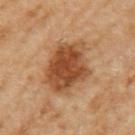Q: Was this lesion biopsied?
A: total-body-photography surveillance lesion; no biopsy
Q: Patient demographics?
A: female, approximately 60 years of age
Q: What is the anatomic site?
A: the left upper arm
Q: Lesion size?
A: about 6.5 mm
Q: What is the imaging modality?
A: total-body-photography crop, ~15 mm field of view
Q: Automated lesion metrics?
A: border irregularity of about 2.5 on a 0–10 scale, internal color variation of about 5 on a 0–10 scale, and peripheral color asymmetry of about 1.5
Q: What lighting was used for the tile?
A: cross-polarized